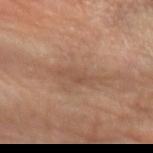Q: Was this lesion biopsied?
A: catalogued during a skin exam; not biopsied
Q: Patient demographics?
A: male, about 60 years old
Q: What did automated image analysis measure?
A: a lesion-detection confidence of about 80/100
Q: What kind of image is this?
A: total-body-photography crop, ~15 mm field of view
Q: What is the anatomic site?
A: the left forearm
Q: How was the tile lit?
A: cross-polarized